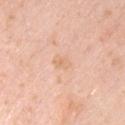follow-up: total-body-photography surveillance lesion; no biopsy
acquisition: ~15 mm crop, total-body skin-cancer survey
automated metrics: a lesion area of about 3.5 mm², an eccentricity of roughly 0.75, and a shape-asymmetry score of about 0.2 (0 = symmetric); a mean CIELAB color near L≈72 a*≈20 b*≈34 and a normalized border contrast of about 4.5; a border-irregularity index near 2/10 and peripheral color asymmetry of about 1.5
lesion diameter: ~2.5 mm (longest diameter)
lighting: white-light
location: the chest
subject: male, aged around 50